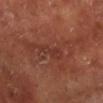The lesion was tiled from a total-body skin photograph and was not biopsied. The recorded lesion diameter is about 4 mm. The lesion is located on the left lower leg. Automated image analysis of the tile measured a footprint of about 4.5 mm², a shape eccentricity near 0.95, and two-axis asymmetry of about 0.35. A male patient in their mid-50s. This image is a 15 mm lesion crop taken from a total-body photograph. This is a cross-polarized tile.